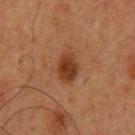<tbp_lesion>
  <biopsy_status>not biopsied; imaged during a skin examination</biopsy_status>
  <patient>
    <sex>male</sex>
    <age_approx>60</age_approx>
  </patient>
  <site>upper back</site>
  <lesion_size>
    <long_diameter_mm_approx>4.0</long_diameter_mm_approx>
  </lesion_size>
  <lighting>cross-polarized</lighting>
  <image>
    <source>total-body photography crop</source>
    <field_of_view_mm>15</field_of_view_mm>
  </image>
</tbp_lesion>